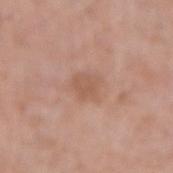notes: imaged on a skin check; not biopsied | tile lighting: white-light illumination | patient: male, in their mid-50s | imaging modality: 15 mm crop, total-body photography | site: the right forearm | TBP lesion metrics: an average lesion color of about L≈56 a*≈20 b*≈29 (CIELAB); a border-irregularity index near 2.5/10, a color-variation rating of about 2/10, and radial color variation of about 0.5.